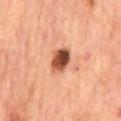  biopsy_status: not biopsied; imaged during a skin examination
  site: abdomen
  patient:
    sex: male
    age_approx: 65
  lesion_size:
    long_diameter_mm_approx: 3.5
  image:
    source: total-body photography crop
    field_of_view_mm: 15
  lighting: cross-polarized
  automated_metrics:
    cielab_L: 40
    cielab_a: 22
    cielab_b: 28
    vs_skin_darker_L: 17.0